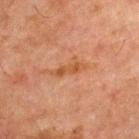notes — total-body-photography surveillance lesion; no biopsy
lighting — cross-polarized
image-analysis metrics — an average lesion color of about L≈43 a*≈22 b*≈32 (CIELAB), about 6 CIELAB-L* units darker than the surrounding skin, and a normalized border contrast of about 6.5; a border-irregularity index near 6/10, a color-variation rating of about 1/10, and peripheral color asymmetry of about 0; a nevus-likeness score of about 0/100 and a detector confidence of about 100 out of 100 that the crop contains a lesion
patient — male, in their mid-60s
lesion size — about 4 mm
site — the chest
image source — total-body-photography crop, ~15 mm field of view